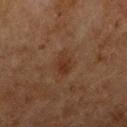The lesion was photographed on a routine skin check and not biopsied; there is no pathology result. A female patient aged 58–62. A lesion tile, about 15 mm wide, cut from a 3D total-body photograph. The recorded lesion diameter is about 3 mm. The lesion is on the arm. Imaged with cross-polarized lighting.